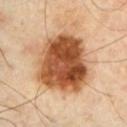<record>
  <biopsy_status>not biopsied; imaged during a skin examination</biopsy_status>
  <site>chest</site>
  <patient>
    <sex>male</sex>
    <age_approx>65</age_approx>
  </patient>
  <image>
    <source>total-body photography crop</source>
    <field_of_view_mm>15</field_of_view_mm>
  </image>
  <lesion_size>
    <long_diameter_mm_approx>7.5</long_diameter_mm_approx>
  </lesion_size>
  <lighting>cross-polarized</lighting>
  <automated_metrics>
    <border_irregularity_0_10>2.0</border_irregularity_0_10>
    <peripheral_color_asymmetry>3.0</peripheral_color_asymmetry>
  </automated_metrics>
</record>